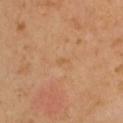biopsy status: imaged on a skin check; not biopsied
lesion diameter: ≈1.5 mm
illumination: cross-polarized
subject: female, aged 33–37
location: the upper back
image source: ~15 mm tile from a whole-body skin photo